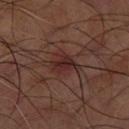The lesion was tiled from a total-body skin photograph and was not biopsied.
A roughly 15 mm field-of-view crop from a total-body skin photograph.
Imaged with cross-polarized lighting.
The subject is a male approximately 65 years of age.
From the arm.